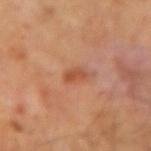Clinical impression:
The lesion was tiled from a total-body skin photograph and was not biopsied.
Context:
From the left forearm. A close-up tile cropped from a whole-body skin photograph, about 15 mm across. Captured under cross-polarized illumination. A female subject, in their 60s.